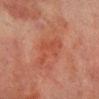{"image": {"source": "total-body photography crop", "field_of_view_mm": 15}, "site": "right lower leg", "lighting": "cross-polarized", "patient": {"sex": "male", "age_approx": 70}, "lesion_size": {"long_diameter_mm_approx": 5.0}}